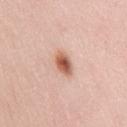biopsy status = total-body-photography surveillance lesion; no biopsy.